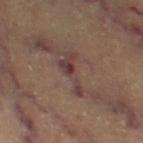workup = total-body-photography surveillance lesion; no biopsy | body site = the left thigh | imaging modality = ~15 mm tile from a whole-body skin photo | patient = aged 58–62.